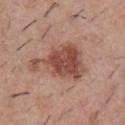Q: Lesion location?
A: the front of the torso
Q: How was the tile lit?
A: white-light illumination
Q: Patient demographics?
A: male, about 30 years old
Q: What kind of image is this?
A: 15 mm crop, total-body photography
Q: What did automated image analysis measure?
A: a shape eccentricity near 0.8 and a symmetry-axis asymmetry near 0.5